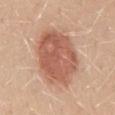Recorded during total-body skin imaging; not selected for excision or biopsy. A roughly 15 mm field-of-view crop from a total-body skin photograph. This is a white-light tile. From the mid back. A female subject, approximately 45 years of age.